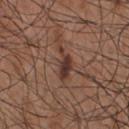biopsy status = no biopsy performed (imaged during a skin exam) | subject = male, aged around 55 | imaging modality = 15 mm crop, total-body photography | automated metrics = a footprint of about 5.5 mm² and an eccentricity of roughly 0.9; a lesion color around L≈36 a*≈19 b*≈24 in CIELAB and a normalized lesion–skin contrast near 9.5 | diameter = ~4 mm (longest diameter) | location = the chest | illumination = white-light.